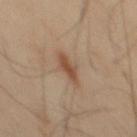Clinical impression:
Part of a total-body skin-imaging series; this lesion was reviewed on a skin check and was not flagged for biopsy.
Context:
The patient is a male aged 43–47. The lesion's longest dimension is about 3.5 mm. The lesion-visualizer software estimated an average lesion color of about L≈48 a*≈18 b*≈30 (CIELAB), about 9 CIELAB-L* units darker than the surrounding skin, and a normalized border contrast of about 7.5. The analysis additionally found a border-irregularity index near 3/10 and peripheral color asymmetry of about 0.5. A region of skin cropped from a whole-body photographic capture, roughly 15 mm wide. Located on the back.